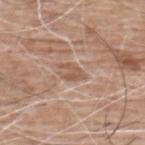No biopsy was performed on this lesion — it was imaged during a full skin examination and was not determined to be concerning. Cropped from a whole-body photographic skin survey; the tile spans about 15 mm. A male patient in their 60s. On the upper back.